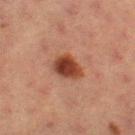Clinical impression: No biopsy was performed on this lesion — it was imaged during a full skin examination and was not determined to be concerning. Image and clinical context: Cropped from a whole-body photographic skin survey; the tile spans about 15 mm. A female patient, approximately 55 years of age. Approximately 3.5 mm at its widest. Captured under cross-polarized illumination. From the left thigh. The total-body-photography lesion software estimated a footprint of about 7.5 mm², an outline eccentricity of about 0.7 (0 = round, 1 = elongated), and a shape-asymmetry score of about 0.2 (0 = symmetric). And it measured a border-irregularity rating of about 2/10, a within-lesion color-variation index near 4/10, and peripheral color asymmetry of about 1.5. It also reported a lesion-detection confidence of about 100/100.